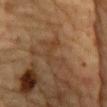{
  "biopsy_status": "not biopsied; imaged during a skin examination",
  "lesion_size": {
    "long_diameter_mm_approx": 4.0
  },
  "image": {
    "source": "total-body photography crop",
    "field_of_view_mm": 15
  },
  "site": "chest",
  "patient": {
    "sex": "male",
    "age_approx": "85+"
  }
}